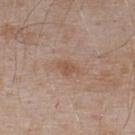follow-up = total-body-photography surveillance lesion; no biopsy
illumination = white-light
lesion diameter = about 2.5 mm
body site = the back
acquisition = 15 mm crop, total-body photography
subject = male, in their mid- to late 50s
image-analysis metrics = a footprint of about 3 mm², a shape eccentricity near 0.8, and two-axis asymmetry of about 0.25